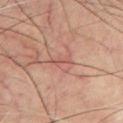This lesion was catalogued during total-body skin photography and was not selected for biopsy. From the chest. Longest diameter approximately 2.5 mm. Automated tile analysis of the lesion measured a lesion–skin lightness drop of about 7 and a lesion-to-skin contrast of about 5 (normalized; higher = more distinct). A roughly 15 mm field-of-view crop from a total-body skin photograph. A male subject aged 58 to 62.